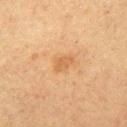biopsy_status: not biopsied; imaged during a skin examination
site: left upper arm
image:
  source: total-body photography crop
  field_of_view_mm: 15
lighting: cross-polarized
patient:
  sex: female
  age_approx: 55
lesion_size:
  long_diameter_mm_approx: 2.5
automated_metrics:
  area_mm2_approx: 4.0
  eccentricity: 0.7
  shape_asymmetry: 0.25
  nevus_likeness_0_100: 5